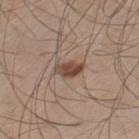{
  "biopsy_status": "not biopsied; imaged during a skin examination",
  "lesion_size": {
    "long_diameter_mm_approx": 3.5
  },
  "image": {
    "source": "total-body photography crop",
    "field_of_view_mm": 15
  },
  "lighting": "white-light",
  "site": "left thigh",
  "patient": {
    "sex": "male",
    "age_approx": 60
  }
}